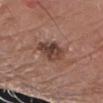notes — total-body-photography surveillance lesion; no biopsy
body site — the right forearm
lighting — white-light
subject — male, about 80 years old
acquisition — 15 mm crop, total-body photography
TBP lesion metrics — a nevus-likeness score of about 25/100 and lesion-presence confidence of about 100/100